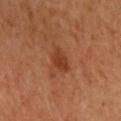Impression: This lesion was catalogued during total-body skin photography and was not selected for biopsy. Acquisition and patient details: A 15 mm close-up extracted from a 3D total-body photography capture. The subject is a male aged approximately 70. Captured under cross-polarized illumination. The lesion is on the back. The recorded lesion diameter is about 3.5 mm.